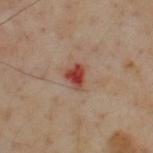biopsy status = total-body-photography surveillance lesion; no biopsy
subject = male, approximately 55 years of age
lesion diameter = ≈2.5 mm
automated lesion analysis = border irregularity of about 3 on a 0–10 scale and a within-lesion color-variation index near 4/10
anatomic site = the upper back
illumination = cross-polarized
imaging modality = ~15 mm crop, total-body skin-cancer survey